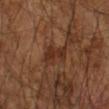Image and clinical context: The lesion's longest dimension is about 4 mm. From the left arm. A 15 mm close-up extracted from a 3D total-body photography capture. A male patient, aged 63 to 67. The total-body-photography lesion software estimated a lesion area of about 7 mm², a shape eccentricity near 0.85, and a shape-asymmetry score of about 0.45 (0 = symmetric).